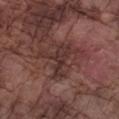Part of a total-body skin-imaging series; this lesion was reviewed on a skin check and was not flagged for biopsy.
Imaged with white-light lighting.
A roughly 15 mm field-of-view crop from a total-body skin photograph.
A male patient, approximately 75 years of age.
Located on the left forearm.